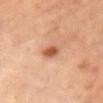This lesion was catalogued during total-body skin photography and was not selected for biopsy.
A female subject, about 60 years old.
Longest diameter approximately 2.5 mm.
Captured under cross-polarized illumination.
The lesion is located on the chest.
Automated tile analysis of the lesion measured a footprint of about 4 mm², an eccentricity of roughly 0.8, and a shape-asymmetry score of about 0.2 (0 = symmetric). The software also gave a lesion–skin lightness drop of about 13. And it measured a border-irregularity rating of about 2/10 and peripheral color asymmetry of about 1. The analysis additionally found a nevus-likeness score of about 90/100 and lesion-presence confidence of about 100/100.
Cropped from a whole-body photographic skin survey; the tile spans about 15 mm.